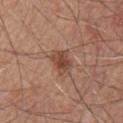• follow-up — imaged on a skin check; not biopsied
• imaging modality — total-body-photography crop, ~15 mm field of view
• patient — male, about 65 years old
• TBP lesion metrics — a mean CIELAB color near L≈45 a*≈21 b*≈28 and a lesion–skin lightness drop of about 11; an automated nevus-likeness rating near 80 out of 100 and a detector confidence of about 100 out of 100 that the crop contains a lesion
• lighting — white-light illumination
• anatomic site — the chest
• size — about 3 mm